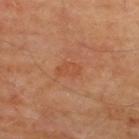body site=the upper back | lesion size=~3 mm (longest diameter) | lighting=cross-polarized | subject=male, in their mid-60s | acquisition=~15 mm tile from a whole-body skin photo.